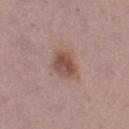Clinical summary:
A female patient aged 18 to 22. On the leg. This image is a 15 mm lesion crop taken from a total-body photograph.